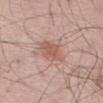Assessment:
Captured during whole-body skin photography for melanoma surveillance; the lesion was not biopsied.
Context:
A lesion tile, about 15 mm wide, cut from a 3D total-body photograph. A male patient aged approximately 60. Captured under white-light illumination. Located on the abdomen.